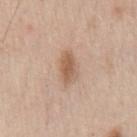The lesion was photographed on a routine skin check and not biopsied; there is no pathology result.
A close-up tile cropped from a whole-body skin photograph, about 15 mm across.
Longest diameter approximately 4 mm.
The lesion is on the chest.
The tile uses white-light illumination.
The subject is a male roughly 70 years of age.